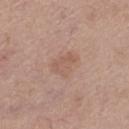Findings:
- follow-up — imaged on a skin check; not biopsied
- tile lighting — white-light
- lesion diameter — ~3.5 mm (longest diameter)
- body site — the left thigh
- image source — 15 mm crop, total-body photography
- patient — female, about 55 years old